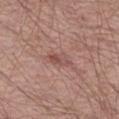This lesion was catalogued during total-body skin photography and was not selected for biopsy. The tile uses white-light illumination. The lesion is located on the left thigh. The subject is a male about 65 years old. This image is a 15 mm lesion crop taken from a total-body photograph. The lesion's longest dimension is about 3 mm.